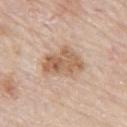This lesion was catalogued during total-body skin photography and was not selected for biopsy. About 5 mm across. Imaged with white-light lighting. A male patient, approximately 80 years of age. This image is a 15 mm lesion crop taken from a total-body photograph. The lesion-visualizer software estimated a border-irregularity rating of about 2.5/10 and peripheral color asymmetry of about 1.5. Located on the left thigh.